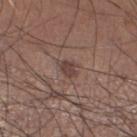The lesion was photographed on a routine skin check and not biopsied; there is no pathology result.
Located on the left lower leg.
An algorithmic analysis of the crop reported internal color variation of about 1.5 on a 0–10 scale and peripheral color asymmetry of about 0.5.
A male subject approximately 55 years of age.
Captured under white-light illumination.
A 15 mm close-up extracted from a 3D total-body photography capture.